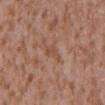Notes:
* follow-up · no biopsy performed (imaged during a skin exam)
* diameter · ≈3 mm
* body site · the mid back
* subject · male, about 45 years old
* image source · ~15 mm tile from a whole-body skin photo
* TBP lesion metrics · an area of roughly 3.5 mm², a shape eccentricity near 0.8, and two-axis asymmetry of about 0.4; a border-irregularity rating of about 4/10 and internal color variation of about 2.5 on a 0–10 scale